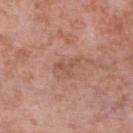Acquisition and patient details: Cropped from a whole-body photographic skin survey; the tile spans about 15 mm. A male subject in their mid- to late 50s. From the right lower leg. This is a white-light tile. The lesion-visualizer software estimated an area of roughly 2.5 mm² and two-axis asymmetry of about 0.6. It also reported a border-irregularity rating of about 7/10, internal color variation of about 0 on a 0–10 scale, and peripheral color asymmetry of about 0. The software also gave an automated nevus-likeness rating near 0 out of 100 and lesion-presence confidence of about 100/100. The recorded lesion diameter is about 3 mm.